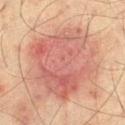Notes:
* biopsy status · total-body-photography surveillance lesion; no biopsy
* body site · the leg
* size · ≈9.5 mm
* automated metrics · a footprint of about 49 mm² and a shape-asymmetry score of about 0.25 (0 = symmetric); an average lesion color of about L≈51 a*≈23 b*≈25 (CIELAB), about 9 CIELAB-L* units darker than the surrounding skin, and a normalized border contrast of about 7
* acquisition · ~15 mm crop, total-body skin-cancer survey
* lighting · cross-polarized
* patient · male, in their mid- to late 70s